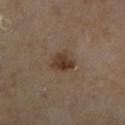Imaged during a routine full-body skin examination; the lesion was not biopsied and no histopathology is available.